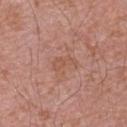This lesion was catalogued during total-body skin photography and was not selected for biopsy.
On the right upper arm.
Cropped from a total-body skin-imaging series; the visible field is about 15 mm.
A male subject aged approximately 50.
Approximately 3 mm at its widest.
Imaged with white-light lighting.